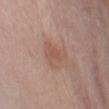{
  "patient": {
    "sex": "female",
    "age_approx": 65
  },
  "lesion_size": {
    "long_diameter_mm_approx": 3.5
  },
  "image": {
    "source": "total-body photography crop",
    "field_of_view_mm": 15
  },
  "lighting": "white-light",
  "automated_metrics": {
    "border_irregularity_0_10": 4.0,
    "nevus_likeness_0_100": 35,
    "lesion_detection_confidence_0_100": 100
  },
  "site": "front of the torso"
}